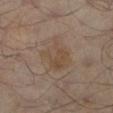biopsy status=catalogued during a skin exam; not biopsied
location=the leg
subject=male, aged approximately 65
TBP lesion metrics=about 5 CIELAB-L* units darker than the surrounding skin and a normalized lesion–skin contrast near 5.5; an automated nevus-likeness rating near 0 out of 100
image source=total-body-photography crop, ~15 mm field of view
lesion size=≈4.5 mm